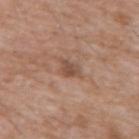Assessment:
Captured during whole-body skin photography for melanoma surveillance; the lesion was not biopsied.
Image and clinical context:
A male subject, aged approximately 60. A lesion tile, about 15 mm wide, cut from a 3D total-body photograph. The total-body-photography lesion software estimated roughly 9 lightness units darker than nearby skin and a normalized border contrast of about 7. And it measured a border-irregularity rating of about 2.5/10, a within-lesion color-variation index near 2/10, and radial color variation of about 0.5. The software also gave a classifier nevus-likeness of about 0/100 and a detector confidence of about 100 out of 100 that the crop contains a lesion. On the front of the torso. The lesion's longest dimension is about 2.5 mm.